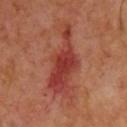Assessment:
Imaged during a routine full-body skin examination; the lesion was not biopsied and no histopathology is available.
Background:
The total-body-photography lesion software estimated an area of roughly 19 mm², an outline eccentricity of about 0.95 (0 = round, 1 = elongated), and a symmetry-axis asymmetry near 0.5. The analysis additionally found an average lesion color of about L≈40 a*≈31 b*≈29 (CIELAB), roughly 10 lightness units darker than nearby skin, and a normalized border contrast of about 8.5. The analysis additionally found a color-variation rating of about 4/10 and radial color variation of about 1. The lesion's longest dimension is about 9 mm. The tile uses cross-polarized illumination. A male patient approximately 65 years of age. A roughly 15 mm field-of-view crop from a total-body skin photograph.